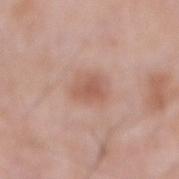Captured during whole-body skin photography for melanoma surveillance; the lesion was not biopsied.